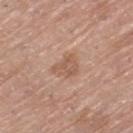Part of a total-body skin-imaging series; this lesion was reviewed on a skin check and was not flagged for biopsy. Captured under white-light illumination. The patient is a female about 65 years old. A roughly 15 mm field-of-view crop from a total-body skin photograph. The lesion is on the left thigh. The recorded lesion diameter is about 3.5 mm.